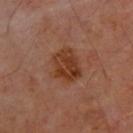Assessment:
Recorded during total-body skin imaging; not selected for excision or biopsy.
Clinical summary:
The lesion is on the upper back. Longest diameter approximately 4 mm. A region of skin cropped from a whole-body photographic capture, roughly 15 mm wide. A male subject about 65 years old. Imaged with cross-polarized lighting. The lesion-visualizer software estimated a lesion–skin lightness drop of about 9 and a normalized lesion–skin contrast near 8.5. And it measured border irregularity of about 2 on a 0–10 scale and a peripheral color-asymmetry measure near 2.